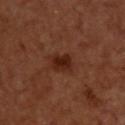Recorded during total-body skin imaging; not selected for excision or biopsy. The lesion-visualizer software estimated a lesion area of about 5.5 mm², a shape eccentricity near 0.65, and a shape-asymmetry score of about 0.25 (0 = symmetric). A roughly 15 mm field-of-view crop from a total-body skin photograph. On the upper back. About 3 mm across. This is a cross-polarized tile.